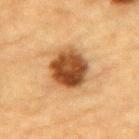Clinical impression:
Part of a total-body skin-imaging series; this lesion was reviewed on a skin check and was not flagged for biopsy.
Image and clinical context:
A male patient in their mid-80s or older. On the mid back. A lesion tile, about 15 mm wide, cut from a 3D total-body photograph.